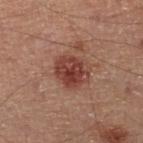biopsy_status: not biopsied; imaged during a skin examination
automated_metrics:
  vs_skin_contrast_norm: 9.0
patient:
  sex: male
  age_approx: 50
lighting: cross-polarized
image:
  source: total-body photography crop
  field_of_view_mm: 15
site: leg
lesion_size:
  long_diameter_mm_approx: 4.0